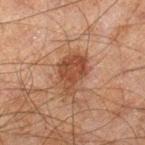A male subject aged 43 to 47. Imaged with cross-polarized lighting. Cropped from a total-body skin-imaging series; the visible field is about 15 mm. The lesion is located on the right lower leg. Approximately 5 mm at its widest.